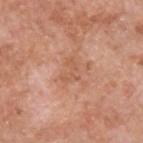subject: male, aged around 60
tile lighting: white-light
diameter: about 3 mm
TBP lesion metrics: a lesion color around L≈57 a*≈24 b*≈33 in CIELAB and a lesion–skin lightness drop of about 7; border irregularity of about 7.5 on a 0–10 scale and radial color variation of about 0; a classifier nevus-likeness of about 0/100 and lesion-presence confidence of about 100/100
body site: the upper back
image: ~15 mm tile from a whole-body skin photo